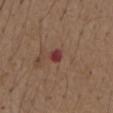Impression: Part of a total-body skin-imaging series; this lesion was reviewed on a skin check and was not flagged for biopsy. Background: The recorded lesion diameter is about 2 mm. The subject is a male roughly 75 years of age. The lesion is on the abdomen. Automated tile analysis of the lesion measured a lesion–skin lightness drop of about 11 and a normalized lesion–skin contrast near 10. The analysis additionally found a detector confidence of about 100 out of 100 that the crop contains a lesion. A region of skin cropped from a whole-body photographic capture, roughly 15 mm wide.